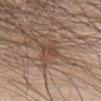| feature | finding |
|---|---|
| image | 15 mm crop, total-body photography |
| location | the chest |
| patient | male, aged 38–42 |
| lighting | white-light illumination |
| size | ≈3 mm |